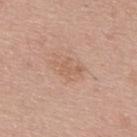subject = male, in their mid-40s; site = the upper back; lesion diameter = about 3.5 mm; tile lighting = white-light illumination; acquisition = ~15 mm crop, total-body skin-cancer survey.